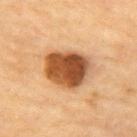{
  "biopsy_status": "not biopsied; imaged during a skin examination",
  "site": "chest",
  "patient": {
    "sex": "male",
    "age_approx": "85+"
  },
  "automated_metrics": {
    "area_mm2_approx": 20.0,
    "eccentricity": 0.6,
    "shape_asymmetry": 0.2
  },
  "lesion_size": {
    "long_diameter_mm_approx": 5.5
  },
  "lighting": "cross-polarized",
  "image": {
    "source": "total-body photography crop",
    "field_of_view_mm": 15
  }
}